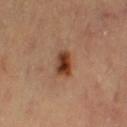follow-up: no biopsy performed (imaged during a skin exam); imaging modality: ~15 mm tile from a whole-body skin photo; diameter: about 3 mm; site: the leg; lighting: cross-polarized; patient: female, aged around 65.